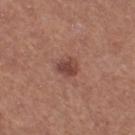This lesion was catalogued during total-body skin photography and was not selected for biopsy. The patient is a female roughly 50 years of age. Approximately 2.5 mm at its widest. A 15 mm close-up tile from a total-body photography series done for melanoma screening. The lesion is located on the right thigh. The tile uses white-light illumination. Automated tile analysis of the lesion measured a lesion color around L≈44 a*≈23 b*≈25 in CIELAB, about 10 CIELAB-L* units darker than the surrounding skin, and a normalized border contrast of about 8. It also reported a border-irregularity index near 2/10, a color-variation rating of about 3.5/10, and radial color variation of about 1. And it measured an automated nevus-likeness rating near 75 out of 100 and a detector confidence of about 100 out of 100 that the crop contains a lesion.